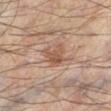The lesion was tiled from a total-body skin photograph and was not biopsied. A roughly 15 mm field-of-view crop from a total-body skin photograph. From the leg. Automated image analysis of the tile measured an area of roughly 5.5 mm², a shape eccentricity near 0.6, and a symmetry-axis asymmetry near 0.4. The analysis additionally found a mean CIELAB color near L≈49 a*≈19 b*≈28, roughly 9 lightness units darker than nearby skin, and a lesion-to-skin contrast of about 6.5 (normalized; higher = more distinct). This is a cross-polarized tile. A male patient, approximately 55 years of age. Measured at roughly 3 mm in maximum diameter.